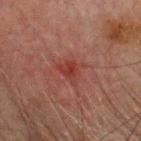Impression:
No biopsy was performed on this lesion — it was imaged during a full skin examination and was not determined to be concerning.
Clinical summary:
A male subject, aged approximately 65. The lesion-visualizer software estimated a lesion area of about 5.5 mm², an outline eccentricity of about 0.65 (0 = round, 1 = elongated), and two-axis asymmetry of about 0.5. The software also gave an automated nevus-likeness rating near 0 out of 100 and lesion-presence confidence of about 100/100. The lesion's longest dimension is about 3.5 mm. Located on the head or neck. Captured under cross-polarized illumination. A 15 mm close-up extracted from a 3D total-body photography capture.